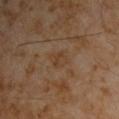Q: Is there a histopathology result?
A: no biopsy performed (imaged during a skin exam)
Q: How was this image acquired?
A: ~15 mm tile from a whole-body skin photo
Q: Patient demographics?
A: male, aged around 60
Q: Lesion size?
A: ~3 mm (longest diameter)
Q: Lesion location?
A: the arm